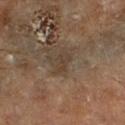A close-up tile cropped from a whole-body skin photograph, about 15 mm across.
A male patient aged around 70.
The lesion is on the right lower leg.